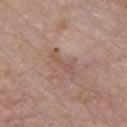* notes — total-body-photography surveillance lesion; no biopsy
* patient — male, in their mid-70s
* size — ≈4 mm
* image — ~15 mm crop, total-body skin-cancer survey
* lighting — white-light
* location — the front of the torso